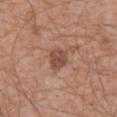No biopsy was performed on this lesion — it was imaged during a full skin examination and was not determined to be concerning.
Longest diameter approximately 2.5 mm.
The lesion is on the mid back.
A 15 mm close-up extracted from a 3D total-body photography capture.
A male patient, aged around 60.